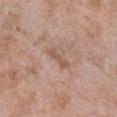Recorded during total-body skin imaging; not selected for excision or biopsy. The lesion is located on the front of the torso. A 15 mm close-up extracted from a 3D total-body photography capture. A female patient aged 73 to 77.